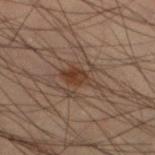A close-up tile cropped from a whole-body skin photograph, about 15 mm across. A male patient, aged 53–57. The recorded lesion diameter is about 3.5 mm. Imaged with cross-polarized lighting. Located on the leg.